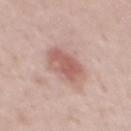biopsy_status: not biopsied; imaged during a skin examination
lesion_size:
  long_diameter_mm_approx: 5.0
patient:
  sex: male
  age_approx: 45
site: mid back
image:
  source: total-body photography crop
  field_of_view_mm: 15
lighting: white-light
automated_metrics:
  cielab_L: 60
  cielab_a: 22
  cielab_b: 24
  vs_skin_darker_L: 11.0
  border_irregularity_0_10: 2.0
  color_variation_0_10: 4.0
  peripheral_color_asymmetry: 1.5
  nevus_likeness_0_100: 80
  lesion_detection_confidence_0_100: 100